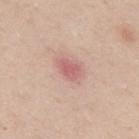workup: imaged on a skin check; not biopsied | location: the upper back | lesion diameter: ~3 mm (longest diameter) | tile lighting: white-light illumination | image source: 15 mm crop, total-body photography | TBP lesion metrics: a lesion area of about 5 mm², an outline eccentricity of about 0.75 (0 = round, 1 = elongated), and two-axis asymmetry of about 0.2; an average lesion color of about L≈61 a*≈25 b*≈23 (CIELAB), roughly 10 lightness units darker than nearby skin, and a normalized border contrast of about 6.5; a classifier nevus-likeness of about 0/100 and a detector confidence of about 100 out of 100 that the crop contains a lesion | patient: female, aged around 30.